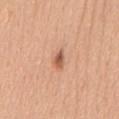Assessment:
Recorded during total-body skin imaging; not selected for excision or biopsy.
Image and clinical context:
Cropped from a total-body skin-imaging series; the visible field is about 15 mm. The patient is a male about 40 years old. Located on the mid back.